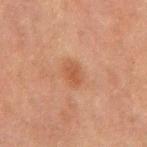Captured during whole-body skin photography for melanoma surveillance; the lesion was not biopsied.
A region of skin cropped from a whole-body photographic capture, roughly 15 mm wide.
The tile uses cross-polarized illumination.
A male patient in their mid- to late 70s.
Located on the mid back.
Longest diameter approximately 3 mm.